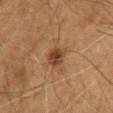Recorded during total-body skin imaging; not selected for excision or biopsy. A 15 mm close-up extracted from a 3D total-body photography capture. The tile uses cross-polarized illumination. The lesion's longest dimension is about 3 mm. A male patient, in their mid-60s. The lesion is located on the chest.